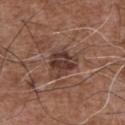biopsy status: total-body-photography surveillance lesion; no biopsy
image source: 15 mm crop, total-body photography
site: the chest
subject: male, aged 73–77